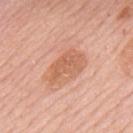workup: total-body-photography surveillance lesion; no biopsy | body site: the upper back | size: ≈5 mm | image-analysis metrics: a footprint of about 12 mm², a shape eccentricity near 0.8, and a symmetry-axis asymmetry near 0.25; an average lesion color of about L≈62 a*≈24 b*≈34 (CIELAB), roughly 9 lightness units darker than nearby skin, and a lesion-to-skin contrast of about 6.5 (normalized; higher = more distinct); a border-irregularity rating of about 2.5/10; a classifier nevus-likeness of about 15/100 and a detector confidence of about 100 out of 100 that the crop contains a lesion | image source: 15 mm crop, total-body photography | tile lighting: white-light illumination | subject: male, aged approximately 80.